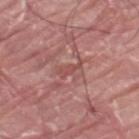The lesion was tiled from a total-body skin photograph and was not biopsied. The lesion is on the left thigh. A male subject, about 40 years old. Measured at roughly 3 mm in maximum diameter. A roughly 15 mm field-of-view crop from a total-body skin photograph.